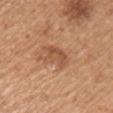Clinical impression:
The lesion was photographed on a routine skin check and not biopsied; there is no pathology result.
Clinical summary:
Captured under white-light illumination. An algorithmic analysis of the crop reported a lesion color around L≈53 a*≈23 b*≈34 in CIELAB, roughly 9 lightness units darker than nearby skin, and a lesion-to-skin contrast of about 6 (normalized; higher = more distinct). The software also gave lesion-presence confidence of about 100/100. From the right upper arm. A lesion tile, about 15 mm wide, cut from a 3D total-body photograph. A female patient aged approximately 55.